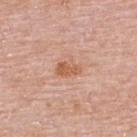The lesion was photographed on a routine skin check and not biopsied; there is no pathology result.
A 15 mm close-up tile from a total-body photography series done for melanoma screening.
Captured under white-light illumination.
Located on the back.
The recorded lesion diameter is about 3 mm.
A female patient about 65 years old.
An algorithmic analysis of the crop reported an area of roughly 5 mm² and two-axis asymmetry of about 0.25. And it measured a mean CIELAB color near L≈60 a*≈24 b*≈34 and a normalized lesion–skin contrast near 7. It also reported a border-irregularity rating of about 2.5/10 and a peripheral color-asymmetry measure near 1. It also reported a nevus-likeness score of about 15/100.